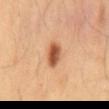Clinical impression: Recorded during total-body skin imaging; not selected for excision or biopsy. Acquisition and patient details: Automated tile analysis of the lesion measured a shape eccentricity near 0.8 and a symmetry-axis asymmetry near 0.25. It also reported lesion-presence confidence of about 100/100. On the mid back. A male subject, in their mid- to late 50s. This is a cross-polarized tile. The recorded lesion diameter is about 3.5 mm. A 15 mm close-up tile from a total-body photography series done for melanoma screening.